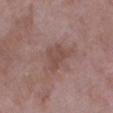Findings:
- follow-up · catalogued during a skin exam; not biopsied
- illumination · white-light illumination
- automated metrics · a lesion area of about 6 mm², an outline eccentricity of about 0.5 (0 = round, 1 = elongated), and two-axis asymmetry of about 0.4; a classifier nevus-likeness of about 0/100
- subject · female, aged approximately 70
- diameter · about 3 mm
- imaging modality · ~15 mm crop, total-body skin-cancer survey
- anatomic site · the leg